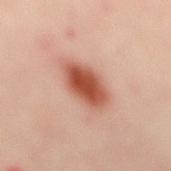biopsy_status: not biopsied; imaged during a skin examination
patient:
  sex: female
  age_approx: 40
image:
  source: total-body photography crop
  field_of_view_mm: 15
site: back
automated_metrics:
  border_irregularity_0_10: 2.5
  color_variation_0_10: 5.5
  peripheral_color_asymmetry: 1.5